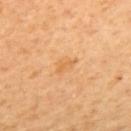biopsy_status: not biopsied; imaged during a skin examination
image:
  source: total-body photography crop
  field_of_view_mm: 15
patient:
  sex: female
  age_approx: 55
site: back